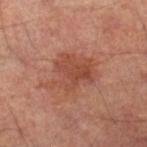| feature | finding |
|---|---|
| biopsy status | no biopsy performed (imaged during a skin exam) |
| patient | male, in their mid- to late 60s |
| image | 15 mm crop, total-body photography |
| anatomic site | the right lower leg |